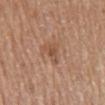image:
  source: total-body photography crop
  field_of_view_mm: 15
lighting: white-light
automated_metrics:
  cielab_L: 51
  cielab_a: 21
  cielab_b: 31
  vs_skin_darker_L: 8.0
  vs_skin_contrast_norm: 6.0
  border_irregularity_0_10: 5.5
  color_variation_0_10: 1.5
  peripheral_color_asymmetry: 0.5
  nevus_likeness_0_100: 5
  lesion_detection_confidence_0_100: 100
patient:
  sex: female
  age_approx: 70
site: right upper arm